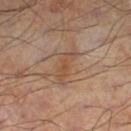biopsy_status: not biopsied; imaged during a skin examination
image:
  source: total-body photography crop
  field_of_view_mm: 15
lesion_size:
  long_diameter_mm_approx: 4.5
site: left lower leg
lighting: cross-polarized
automated_metrics:
  nevus_likeness_0_100: 0
  lesion_detection_confidence_0_100: 100
patient:
  sex: male
  age_approx: 55